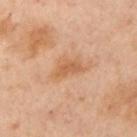• follow-up · total-body-photography surveillance lesion; no biopsy
• lesion diameter · about 4 mm
• site · the chest
• tile lighting · cross-polarized
• patient · female, about 55 years old
• acquisition · ~15 mm tile from a whole-body skin photo